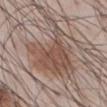<case>
  <biopsy_status>not biopsied; imaged during a skin examination</biopsy_status>
  <lesion_size>
    <long_diameter_mm_approx>9.0</long_diameter_mm_approx>
  </lesion_size>
  <site>abdomen</site>
  <patient>
    <sex>male</sex>
    <age_approx>55</age_approx>
  </patient>
  <automated_metrics>
    <area_mm2_approx>30.0</area_mm2_approx>
    <eccentricity>0.65</eccentricity>
    <shape_asymmetry>0.55</shape_asymmetry>
    <cielab_L>51</cielab_L>
    <cielab_a>16</cielab_a>
    <cielab_b>24</cielab_b>
    <vs_skin_darker_L>10.0</vs_skin_darker_L>
    <vs_skin_contrast_norm>7.5</vs_skin_contrast_norm>
    <border_irregularity_0_10>8.0</border_irregularity_0_10>
    <color_variation_0_10>4.0</color_variation_0_10>
  </automated_metrics>
  <image>
    <source>total-body photography crop</source>
    <field_of_view_mm>15</field_of_view_mm>
  </image>
</case>